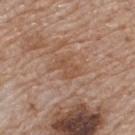workup: imaged on a skin check; not biopsied
lesion size: about 3 mm
body site: the upper back
subject: male, about 80 years old
automated metrics: border irregularity of about 3.5 on a 0–10 scale, a color-variation rating of about 1.5/10, and peripheral color asymmetry of about 0.5; a detector confidence of about 100 out of 100 that the crop contains a lesion
image source: ~15 mm crop, total-body skin-cancer survey
tile lighting: white-light